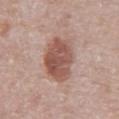lesion size=~5.5 mm (longest diameter) | image source=total-body-photography crop, ~15 mm field of view | illumination=white-light illumination | patient=male, aged 68 to 72 | body site=the abdomen.